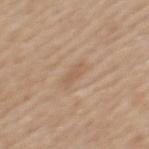{"biopsy_status": "not biopsied; imaged during a skin examination", "site": "back", "patient": {"sex": "female", "age_approx": 50}, "lighting": "white-light", "automated_metrics": {"eccentricity": 0.85, "shape_asymmetry": 0.3, "vs_skin_darker_L": 6.0, "vs_skin_contrast_norm": 4.5, "border_irregularity_0_10": 3.0, "color_variation_0_10": 1.5, "peripheral_color_asymmetry": 0.5}, "image": {"source": "total-body photography crop", "field_of_view_mm": 15}}